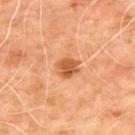Assessment: Recorded during total-body skin imaging; not selected for excision or biopsy. Acquisition and patient details: A close-up tile cropped from a whole-body skin photograph, about 15 mm across. The tile uses cross-polarized illumination. Located on the back. Longest diameter approximately 3 mm. The patient is a male aged 63–67.